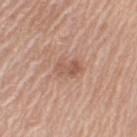biopsy_status: not biopsied; imaged during a skin examination